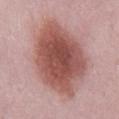Part of a total-body skin-imaging series; this lesion was reviewed on a skin check and was not flagged for biopsy. About 10.5 mm across. A male patient in their mid- to late 50s. The lesion is on the back. A 15 mm crop from a total-body photograph taken for skin-cancer surveillance. This is a white-light tile.